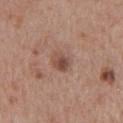Impression:
No biopsy was performed on this lesion — it was imaged during a full skin examination and was not determined to be concerning.
Context:
A 15 mm close-up extracted from a 3D total-body photography capture. Captured under white-light illumination. The patient is a male aged around 65. From the chest. The lesion's longest dimension is about 2.5 mm.